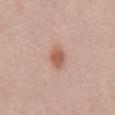notes — imaged on a skin check; not biopsied | automated lesion analysis — a shape eccentricity near 0.7; a color-variation rating of about 2/10 and radial color variation of about 0.5 | diameter — about 3 mm | acquisition — 15 mm crop, total-body photography | location — the mid back | patient — male, approximately 50 years of age.